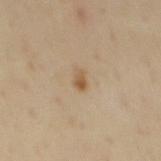Acquisition and patient details: A male patient, aged approximately 55. Located on the mid back. Longest diameter approximately 2 mm. This image is a 15 mm lesion crop taken from a total-body photograph. Imaged with cross-polarized lighting.